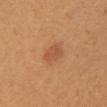Clinical impression:
This lesion was catalogued during total-body skin photography and was not selected for biopsy.
Acquisition and patient details:
Captured under white-light illumination. The lesion is located on the chest. Longest diameter approximately 2.5 mm. A female subject, roughly 25 years of age. Automated image analysis of the tile measured a mean CIELAB color near L≈54 a*≈26 b*≈36, a lesion–skin lightness drop of about 8, and a normalized border contrast of about 5.5. The software also gave an automated nevus-likeness rating near 70 out of 100 and lesion-presence confidence of about 100/100. This image is a 15 mm lesion crop taken from a total-body photograph.